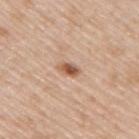Notes:
- biopsy status · total-body-photography surveillance lesion; no biopsy
- image-analysis metrics · a footprint of about 3.5 mm², an outline eccentricity of about 0.8 (0 = round, 1 = elongated), and a symmetry-axis asymmetry near 0.25
- imaging modality · ~15 mm crop, total-body skin-cancer survey
- lesion size · about 2.5 mm
- lighting · white-light
- anatomic site · the upper back
- patient · male, aged approximately 50